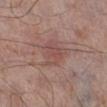Impression: Imaged during a routine full-body skin examination; the lesion was not biopsied and no histopathology is available. Acquisition and patient details: The patient is a male aged around 70. The tile uses white-light illumination. Located on the right lower leg. A region of skin cropped from a whole-body photographic capture, roughly 15 mm wide. The lesion's longest dimension is about 4.5 mm. The total-body-photography lesion software estimated an average lesion color of about L≈49 a*≈21 b*≈22 (CIELAB), roughly 6 lightness units darker than nearby skin, and a lesion-to-skin contrast of about 5 (normalized; higher = more distinct). The analysis additionally found a classifier nevus-likeness of about 0/100 and lesion-presence confidence of about 100/100.